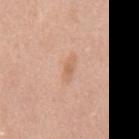Q: What is the anatomic site?
A: the mid back
Q: What are the patient's age and sex?
A: female, approximately 40 years of age
Q: What is the imaging modality?
A: ~15 mm tile from a whole-body skin photo
Q: What lighting was used for the tile?
A: white-light
Q: Lesion size?
A: ~3 mm (longest diameter)